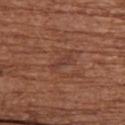Assessment:
No biopsy was performed on this lesion — it was imaged during a full skin examination and was not determined to be concerning.
Acquisition and patient details:
The tile uses white-light illumination. The recorded lesion diameter is about 3 mm. The lesion is located on the back. Cropped from a total-body skin-imaging series; the visible field is about 15 mm. The lesion-visualizer software estimated a classifier nevus-likeness of about 0/100 and a detector confidence of about 85 out of 100 that the crop contains a lesion. A female subject, aged 78 to 82.